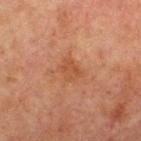Impression:
No biopsy was performed on this lesion — it was imaged during a full skin examination and was not determined to be concerning.
Acquisition and patient details:
A male subject, in their mid-60s. An algorithmic analysis of the crop reported a footprint of about 4 mm², an outline eccentricity of about 0.65 (0 = round, 1 = elongated), and two-axis asymmetry of about 0.25. The analysis additionally found a lesion color around L≈40 a*≈22 b*≈30 in CIELAB, about 6 CIELAB-L* units darker than the surrounding skin, and a lesion-to-skin contrast of about 5.5 (normalized; higher = more distinct). The software also gave a border-irregularity index near 2.5/10 and internal color variation of about 1.5 on a 0–10 scale. The software also gave a nevus-likeness score of about 0/100. On the chest. A lesion tile, about 15 mm wide, cut from a 3D total-body photograph.